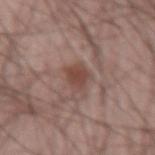Notes:
* notes: imaged on a skin check; not biopsied
* subject: male, about 65 years old
* lesion size: ≈3 mm
* location: the left forearm
* image: 15 mm crop, total-body photography